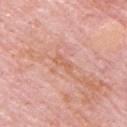Impression: No biopsy was performed on this lesion — it was imaged during a full skin examination and was not determined to be concerning. Background: The lesion's longest dimension is about 3 mm. Imaged with white-light lighting. The subject is a male approximately 65 years of age. From the upper back. A 15 mm close-up extracted from a 3D total-body photography capture.